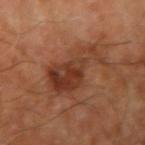Imaged with cross-polarized lighting. Located on the arm. Longest diameter approximately 7.5 mm. This image is a 15 mm lesion crop taken from a total-body photograph. The lesion-visualizer software estimated a lesion color around L≈37 a*≈23 b*≈30 in CIELAB, a lesion–skin lightness drop of about 10, and a lesion-to-skin contrast of about 8 (normalized; higher = more distinct). A male patient in their 70s.